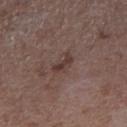– biopsy status · total-body-photography surveillance lesion; no biopsy
– anatomic site · the right forearm
– acquisition · ~15 mm crop, total-body skin-cancer survey
– subject · male, roughly 50 years of age
– lighting · white-light